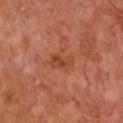Captured under cross-polarized illumination. The subject is a male aged approximately 65. The lesion's longest dimension is about 3 mm. Automated image analysis of the tile measured a nevus-likeness score of about 0/100 and a detector confidence of about 100 out of 100 that the crop contains a lesion. Cropped from a total-body skin-imaging series; the visible field is about 15 mm.